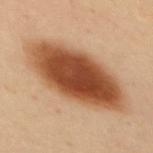notes=catalogued during a skin exam; not biopsied
patient=female, roughly 40 years of age
imaging modality=total-body-photography crop, ~15 mm field of view
anatomic site=the upper back
illumination=cross-polarized